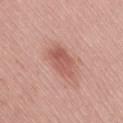Impression: Recorded during total-body skin imaging; not selected for excision or biopsy. Acquisition and patient details: The patient is a female aged 53–57. From the right thigh. Cropped from a total-body skin-imaging series; the visible field is about 15 mm.